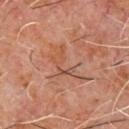Impression: Part of a total-body skin-imaging series; this lesion was reviewed on a skin check and was not flagged for biopsy. Image and clinical context: The lesion is located on the chest. A male subject, aged 58–62. Cropped from a total-body skin-imaging series; the visible field is about 15 mm. Automated tile analysis of the lesion measured roughly 6 lightness units darker than nearby skin and a lesion-to-skin contrast of about 4.5 (normalized; higher = more distinct). It also reported a nevus-likeness score of about 0/100 and a lesion-detection confidence of about 85/100. The recorded lesion diameter is about 6 mm. The tile uses cross-polarized illumination.